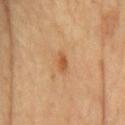Clinical impression: The lesion was tiled from a total-body skin photograph and was not biopsied. Background: Longest diameter approximately 2.5 mm. The tile uses cross-polarized illumination. A male subject, roughly 70 years of age. From the front of the torso. A 15 mm close-up tile from a total-body photography series done for melanoma screening. Automated tile analysis of the lesion measured a lesion area of about 2.5 mm² and an outline eccentricity of about 0.9 (0 = round, 1 = elongated). The software also gave a mean CIELAB color near L≈54 a*≈24 b*≈39 and a normalized border contrast of about 7.5. The analysis additionally found border irregularity of about 2 on a 0–10 scale, a color-variation rating of about 0.5/10, and a peripheral color-asymmetry measure near 0.